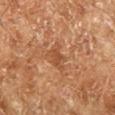Assessment:
This lesion was catalogued during total-body skin photography and was not selected for biopsy.
Context:
A male subject aged around 70. The lesion is on the leg. A 15 mm close-up tile from a total-body photography series done for melanoma screening.